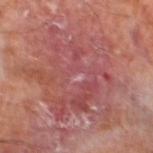Context: The patient is a male about 70 years old. The total-body-photography lesion software estimated an area of roughly 60 mm² and a symmetry-axis asymmetry near 0.3. The software also gave a lesion color around L≈49 a*≈27 b*≈22 in CIELAB and a lesion-to-skin contrast of about 5 (normalized; higher = more distinct). The software also gave a within-lesion color-variation index near 7.5/10. It also reported a classifier nevus-likeness of about 0/100 and a lesion-detection confidence of about 65/100. This is a cross-polarized tile. Approximately 10.5 mm at its widest. From the left lower leg. A lesion tile, about 15 mm wide, cut from a 3D total-body photograph.